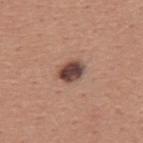notes = catalogued during a skin exam; not biopsied | image-analysis metrics = a lesion color around L≈44 a*≈20 b*≈23 in CIELAB and a lesion–skin lightness drop of about 17; an automated nevus-likeness rating near 80 out of 100 and lesion-presence confidence of about 100/100 | patient = male, aged 43–47 | imaging modality = ~15 mm tile from a whole-body skin photo | site = the mid back | lesion diameter = ~3 mm (longest diameter) | illumination = white-light illumination.